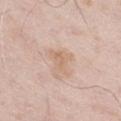Clinical impression:
Imaged during a routine full-body skin examination; the lesion was not biopsied and no histopathology is available.
Acquisition and patient details:
A 15 mm close-up extracted from a 3D total-body photography capture. The tile uses white-light illumination. On the leg. The subject is a male about 60 years old.